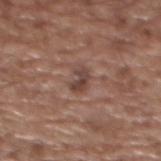Clinical impression:
Captured during whole-body skin photography for melanoma surveillance; the lesion was not biopsied.
Clinical summary:
A lesion tile, about 15 mm wide, cut from a 3D total-body photograph. The subject is a female aged 73 to 77. On the upper back. The recorded lesion diameter is about 3 mm. The tile uses white-light illumination.